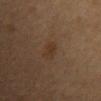notes: imaged on a skin check; not biopsied | tile lighting: cross-polarized illumination | site: the mid back | patient: female, in their mid- to late 50s | image: total-body-photography crop, ~15 mm field of view.